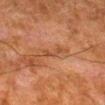notes = imaged on a skin check; not biopsied
subject = male, about 80 years old
anatomic site = the right lower leg
tile lighting = cross-polarized
imaging modality = ~15 mm crop, total-body skin-cancer survey
lesion diameter = ≈3 mm
automated lesion analysis = a lesion area of about 3 mm², a shape eccentricity near 0.95, and a symmetry-axis asymmetry near 0.45; a classifier nevus-likeness of about 0/100 and a lesion-detection confidence of about 90/100